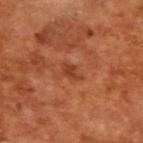Q: How was the tile lit?
A: cross-polarized
Q: What is the lesion's diameter?
A: ≈3 mm
Q: How was this image acquired?
A: 15 mm crop, total-body photography
Q: Who is the patient?
A: male, aged around 65
Q: What did automated image analysis measure?
A: an area of roughly 3 mm², an outline eccentricity of about 0.85 (0 = round, 1 = elongated), and a symmetry-axis asymmetry near 0.4; a border-irregularity index near 4.5/10, internal color variation of about 1 on a 0–10 scale, and a peripheral color-asymmetry measure near 0.5